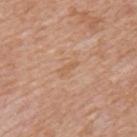  biopsy_status: not biopsied; imaged during a skin examination
  site: mid back
  lesion_size:
    long_diameter_mm_approx: 2.5
  lighting: white-light
  patient:
    sex: male
    age_approx: 60
  image:
    source: total-body photography crop
    field_of_view_mm: 15